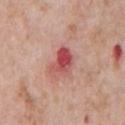Assessment:
Part of a total-body skin-imaging series; this lesion was reviewed on a skin check and was not flagged for biopsy.
Acquisition and patient details:
Longest diameter approximately 4 mm. From the front of the torso. Automated tile analysis of the lesion measured a lesion color around L≈53 a*≈32 b*≈25 in CIELAB and a normalized lesion–skin contrast near 8.5. It also reported border irregularity of about 3.5 on a 0–10 scale and a peripheral color-asymmetry measure near 4. A male subject, about 60 years old. A roughly 15 mm field-of-view crop from a total-body skin photograph. Captured under white-light illumination.